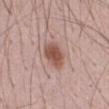Q: Was this lesion biopsied?
A: no biopsy performed (imaged during a skin exam)
Q: How large is the lesion?
A: about 3.5 mm
Q: How was this image acquired?
A: total-body-photography crop, ~15 mm field of view
Q: Where on the body is the lesion?
A: the abdomen
Q: What are the patient's age and sex?
A: male, approximately 60 years of age
Q: What lighting was used for the tile?
A: white-light illumination